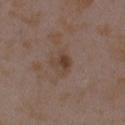| key | value |
|---|---|
| lesion size | ~2.5 mm (longest diameter) |
| image | 15 mm crop, total-body photography |
| automated lesion analysis | an average lesion color of about L≈40 a*≈16 b*≈25 (CIELAB) and a lesion-to-skin contrast of about 7 (normalized; higher = more distinct); a nevus-likeness score of about 25/100 and a lesion-detection confidence of about 100/100 |
| location | the left upper arm |
| illumination | white-light illumination |
| patient | female, aged around 35 |